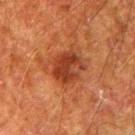Cropped from a whole-body photographic skin survey; the tile spans about 15 mm.
The patient is a male approximately 80 years of age.
Automated tile analysis of the lesion measured a border-irregularity index near 2/10, internal color variation of about 5 on a 0–10 scale, and a peripheral color-asymmetry measure near 1.5.
Captured under cross-polarized illumination.
On the right upper arm.